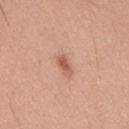workup: catalogued during a skin exam; not biopsied | subject: male, aged 33 to 37 | location: the mid back | lighting: white-light | imaging modality: total-body-photography crop, ~15 mm field of view | size: about 2.5 mm.